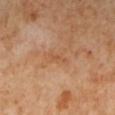| key | value |
|---|---|
| image-analysis metrics | a lesion area of about 1.5 mm², an outline eccentricity of about 0.95 (0 = round, 1 = elongated), and a shape-asymmetry score of about 0.5 (0 = symmetric); an average lesion color of about L≈55 a*≈24 b*≈37 (CIELAB), roughly 6 lightness units darker than nearby skin, and a normalized border contrast of about 5 |
| location | the left lower leg |
| lesion size | about 2.5 mm |
| patient | female, roughly 50 years of age |
| image | ~15 mm tile from a whole-body skin photo |